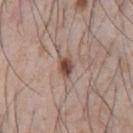This lesion was catalogued during total-body skin photography and was not selected for biopsy. The subject is a male aged approximately 75. The lesion is located on the chest. A 15 mm close-up tile from a total-body photography series done for melanoma screening. The lesion-visualizer software estimated two-axis asymmetry of about 0.2. The analysis additionally found a mean CIELAB color near L≈48 a*≈18 b*≈24, roughly 13 lightness units darker than nearby skin, and a normalized lesion–skin contrast near 9.5. The software also gave a border-irregularity index near 2/10 and internal color variation of about 4.5 on a 0–10 scale. Approximately 2.5 mm at its widest. Captured under white-light illumination.